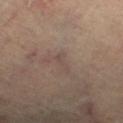| field | value |
|---|---|
| notes | imaged on a skin check; not biopsied |
| anatomic site | the right thigh |
| illumination | cross-polarized |
| diameter | ≈2.5 mm |
| TBP lesion metrics | roughly 5 lightness units darker than nearby skin and a lesion-to-skin contrast of about 5 (normalized; higher = more distinct); a nevus-likeness score of about 0/100 and a lesion-detection confidence of about 75/100 |
| acquisition | ~15 mm crop, total-body skin-cancer survey |
| patient | female, aged 58–62 |